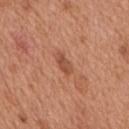biopsy status: imaged on a skin check; not biopsied
location: the back
subject: male, aged approximately 65
acquisition: ~15 mm tile from a whole-body skin photo
image-analysis metrics: an outline eccentricity of about 0.85 (0 = round, 1 = elongated) and a symmetry-axis asymmetry near 0.2; about 9 CIELAB-L* units darker than the surrounding skin
lesion size: ≈2.5 mm